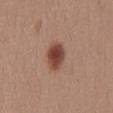{
  "biopsy_status": "not biopsied; imaged during a skin examination",
  "site": "back",
  "patient": {
    "sex": "female",
    "age_approx": 55
  },
  "image": {
    "source": "total-body photography crop",
    "field_of_view_mm": 15
  }
}